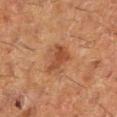<tbp_lesion>
  <biopsy_status>not biopsied; imaged during a skin examination</biopsy_status>
  <lighting>cross-polarized</lighting>
  <image>
    <source>total-body photography crop</source>
    <field_of_view_mm>15</field_of_view_mm>
  </image>
  <lesion_size>
    <long_diameter_mm_approx>3.5</long_diameter_mm_approx>
  </lesion_size>
  <patient>
    <sex>female</sex>
    <age_approx>50</age_approx>
  </patient>
  <site>leg</site>
</tbp_lesion>